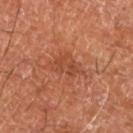This lesion was catalogued during total-body skin photography and was not selected for biopsy. The tile uses cross-polarized illumination. The patient is aged 63 to 67. A 15 mm close-up extracted from a 3D total-body photography capture. Automated image analysis of the tile measured an area of roughly 6.5 mm², a shape eccentricity near 0.75, and a symmetry-axis asymmetry near 0.35. The analysis additionally found a nevus-likeness score of about 5/100 and a detector confidence of about 100 out of 100 that the crop contains a lesion. The lesion is on the left lower leg.